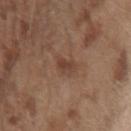Imaged during a routine full-body skin examination; the lesion was not biopsied and no histopathology is available.
The lesion is on the left forearm.
Cropped from a total-body skin-imaging series; the visible field is about 15 mm.
A male subject, approximately 70 years of age.
Automated tile analysis of the lesion measured an area of roughly 3 mm² and a shape-asymmetry score of about 0.25 (0 = symmetric). The software also gave a lesion color around L≈41 a*≈19 b*≈26 in CIELAB, about 8 CIELAB-L* units darker than the surrounding skin, and a lesion-to-skin contrast of about 6.5 (normalized; higher = more distinct). The analysis additionally found a nevus-likeness score of about 0/100 and a detector confidence of about 100 out of 100 that the crop contains a lesion.